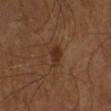Q: Was this lesion biopsied?
A: total-body-photography surveillance lesion; no biopsy
Q: Patient demographics?
A: male, aged around 65
Q: What is the anatomic site?
A: the left leg
Q: What kind of image is this?
A: ~15 mm crop, total-body skin-cancer survey
Q: How was the tile lit?
A: cross-polarized
Q: What did automated image analysis measure?
A: a footprint of about 4 mm², an outline eccentricity of about 0.75 (0 = round, 1 = elongated), and two-axis asymmetry of about 0.35
Q: Lesion size?
A: ~2.5 mm (longest diameter)